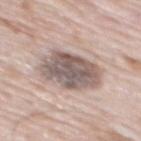Impression: Captured during whole-body skin photography for melanoma surveillance; the lesion was not biopsied. Image and clinical context: This image is a 15 mm lesion crop taken from a total-body photograph. The lesion is located on the back. The lesion-visualizer software estimated a footprint of about 22 mm², an eccentricity of roughly 0.6, and a symmetry-axis asymmetry near 0.15. And it measured about 15 CIELAB-L* units darker than the surrounding skin and a lesion-to-skin contrast of about 10 (normalized; higher = more distinct). It also reported a border-irregularity rating of about 2/10, a within-lesion color-variation index near 5.5/10, and a peripheral color-asymmetry measure near 1.5. About 5.5 mm across. A male patient, aged approximately 80.